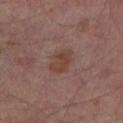Part of a total-body skin-imaging series; this lesion was reviewed on a skin check and was not flagged for biopsy. The lesion's longest dimension is about 3.5 mm. From the left thigh. A 15 mm crop from a total-body photograph taken for skin-cancer surveillance. The subject is a male aged approximately 65.